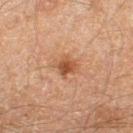workup: imaged on a skin check; not biopsied | subject: male, aged approximately 60 | lesion diameter: ≈3 mm | acquisition: ~15 mm tile from a whole-body skin photo | anatomic site: the leg | illumination: cross-polarized illumination | automated lesion analysis: a lesion color around L≈40 a*≈19 b*≈28 in CIELAB, a lesion–skin lightness drop of about 8, and a normalized border contrast of about 7; a border-irregularity index near 3.5/10, a color-variation rating of about 3.5/10, and a peripheral color-asymmetry measure near 1; a classifier nevus-likeness of about 75/100.